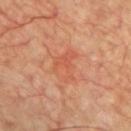Clinical impression: The lesion was photographed on a routine skin check and not biopsied; there is no pathology result. Acquisition and patient details: Approximately 4 mm at its widest. The total-body-photography lesion software estimated a shape eccentricity near 0.7 and a shape-asymmetry score of about 0.55 (0 = symmetric). And it measured lesion-presence confidence of about 100/100. From the chest. Imaged with cross-polarized lighting. Cropped from a total-body skin-imaging series; the visible field is about 15 mm. The subject is a male about 60 years old.